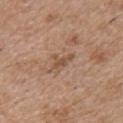The lesion was photographed on a routine skin check and not biopsied; there is no pathology result. Cropped from a total-body skin-imaging series; the visible field is about 15 mm. The lesion is on the chest. The patient is a male about 50 years old. This is a white-light tile.